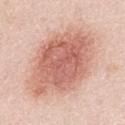Q: Was this lesion biopsied?
A: catalogued during a skin exam; not biopsied
Q: Automated lesion metrics?
A: a lesion color around L≈64 a*≈24 b*≈28 in CIELAB and a normalized lesion–skin contrast near 8; a border-irregularity index near 1.5/10 and radial color variation of about 1.5
Q: Where on the body is the lesion?
A: the front of the torso
Q: Patient demographics?
A: female, in their mid-60s
Q: What is the imaging modality?
A: ~15 mm crop, total-body skin-cancer survey
Q: How large is the lesion?
A: ≈10.5 mm